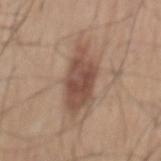A male subject aged 63–67.
The tile uses white-light illumination.
A 15 mm close-up tile from a total-body photography series done for melanoma screening.
The lesion is located on the mid back.